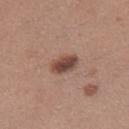Context: An algorithmic analysis of the crop reported a lesion area of about 6 mm². The software also gave border irregularity of about 2 on a 0–10 scale and a color-variation rating of about 4/10. A 15 mm close-up tile from a total-body photography series done for melanoma screening. The lesion is on the right thigh. The tile uses white-light illumination. A female patient, aged around 30. Longest diameter approximately 3.5 mm.